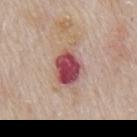Context:
A male patient, aged 63–67. Located on the mid back. A region of skin cropped from a whole-body photographic capture, roughly 15 mm wide.